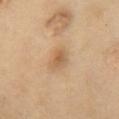No biopsy was performed on this lesion — it was imaged during a full skin examination and was not determined to be concerning. On the chest. Approximately 2.5 mm at its widest. A region of skin cropped from a whole-body photographic capture, roughly 15 mm wide. The patient is a female aged around 55.